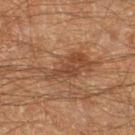notes: catalogued during a skin exam; not biopsied | patient: male, aged around 60 | location: the left thigh | image: ~15 mm crop, total-body skin-cancer survey.